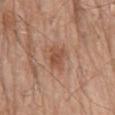Part of a total-body skin-imaging series; this lesion was reviewed on a skin check and was not flagged for biopsy. Measured at roughly 3 mm in maximum diameter. A male subject, about 80 years old. The tile uses white-light illumination. On the mid back. A 15 mm close-up tile from a total-body photography series done for melanoma screening.